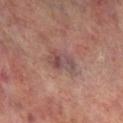Q: Was a biopsy performed?
A: no biopsy performed (imaged during a skin exam)
Q: Where on the body is the lesion?
A: the right lower leg
Q: What lighting was used for the tile?
A: cross-polarized
Q: Lesion size?
A: ~4 mm (longest diameter)
Q: How was this image acquired?
A: 15 mm crop, total-body photography
Q: Who is the patient?
A: male, roughly 70 years of age